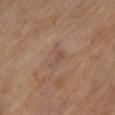{
  "biopsy_status": "not biopsied; imaged during a skin examination",
  "image": {
    "source": "total-body photography crop",
    "field_of_view_mm": 15
  },
  "patient": {
    "sex": "female",
    "age_approx": 70
  },
  "lesion_size": {
    "long_diameter_mm_approx": 3.0
  },
  "site": "right lower leg",
  "lighting": "cross-polarized"
}